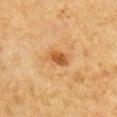  biopsy_status: not biopsied; imaged during a skin examination
  patient:
    sex: male
    age_approx: 65
  automated_metrics:
    nevus_likeness_0_100: 85
    lesion_detection_confidence_0_100: 100
  image:
    source: total-body photography crop
    field_of_view_mm: 15
  site: right upper arm
  lesion_size:
    long_diameter_mm_approx: 2.5
  lighting: cross-polarized